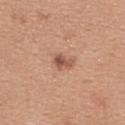  biopsy_status: not biopsied; imaged during a skin examination
  automated_metrics:
    area_mm2_approx: 4.0
    eccentricity: 0.75
    shape_asymmetry: 0.3
    cielab_L: 54
    cielab_a: 22
    cielab_b: 30
    vs_skin_darker_L: 11.0
    border_irregularity_0_10: 3.0
    color_variation_0_10: 5.5
    peripheral_color_asymmetry: 2.0
  patient:
    sex: female
    age_approx: 45
  site: upper back
  image:
    source: total-body photography crop
    field_of_view_mm: 15
  lighting: white-light